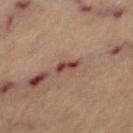<tbp_lesion>
<biopsy_status>not biopsied; imaged during a skin examination</biopsy_status>
<image>
  <source>total-body photography crop</source>
  <field_of_view_mm>15</field_of_view_mm>
</image>
<patient>
  <sex>female</sex>
  <age_approx>65</age_approx>
</patient>
<site>left thigh</site>
<lighting>cross-polarized</lighting>
<automated_metrics>
  <area_mm2_approx>3.0</area_mm2_approx>
  <eccentricity>0.9</eccentricity>
  <shape_asymmetry>0.4</shape_asymmetry>
  <border_irregularity_0_10>3.5</border_irregularity_0_10>
  <peripheral_color_asymmetry>0.0</peripheral_color_asymmetry>
  <nevus_likeness_0_100>0</nevus_likeness_0_100>
  <lesion_detection_confidence_0_100>85</lesion_detection_confidence_0_100>
</automated_metrics>
<lesion_size>
  <long_diameter_mm_approx>2.5</long_diameter_mm_approx>
</lesion_size>
</tbp_lesion>